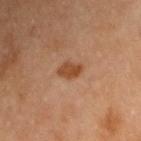<case>
<site>right upper arm</site>
<image>
  <source>total-body photography crop</source>
  <field_of_view_mm>15</field_of_view_mm>
</image>
<patient>
  <sex>female</sex>
  <age_approx>55</age_approx>
</patient>
<lighting>cross-polarized</lighting>
</case>